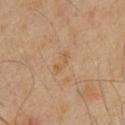Part of a total-body skin-imaging series; this lesion was reviewed on a skin check and was not flagged for biopsy. This is a cross-polarized tile. Approximately 3 mm at its widest. An algorithmic analysis of the crop reported an area of roughly 3 mm² and two-axis asymmetry of about 0.5. And it measured an automated nevus-likeness rating near 0 out of 100 and lesion-presence confidence of about 100/100. A male subject roughly 60 years of age. A 15 mm close-up extracted from a 3D total-body photography capture. On the front of the torso.